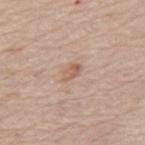Q: Is there a histopathology result?
A: no biopsy performed (imaged during a skin exam)
Q: What did automated image analysis measure?
A: a mean CIELAB color near L≈59 a*≈19 b*≈29, roughly 9 lightness units darker than nearby skin, and a normalized border contrast of about 6.5
Q: Lesion size?
A: ≈2.5 mm
Q: What is the imaging modality?
A: 15 mm crop, total-body photography
Q: Where on the body is the lesion?
A: the mid back
Q: What lighting was used for the tile?
A: white-light
Q: What are the patient's age and sex?
A: male, aged around 75